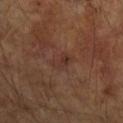follow-up: total-body-photography surveillance lesion; no biopsy | image-analysis metrics: an eccentricity of roughly 0.7 and two-axis asymmetry of about 0.25; a lesion–skin lightness drop of about 5 and a lesion-to-skin contrast of about 5.5 (normalized; higher = more distinct); a nevus-likeness score of about 5/100 and a lesion-detection confidence of about 100/100 | image source: ~15 mm crop, total-body skin-cancer survey | size: about 3 mm | body site: the left forearm | lighting: cross-polarized illumination | subject: male, aged around 65.